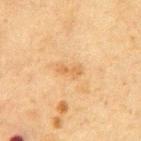Case summary:
– notes: no biopsy performed (imaged during a skin exam)
– patient: male, roughly 75 years of age
– size: ≈3 mm
– image: ~15 mm crop, total-body skin-cancer survey
– body site: the abdomen